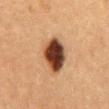| field | value |
|---|---|
| workup | no biopsy performed (imaged during a skin exam) |
| patient | female, aged approximately 60 |
| image | ~15 mm crop, total-body skin-cancer survey |
| site | the chest |